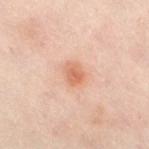Case summary:
• illumination: cross-polarized
• acquisition: total-body-photography crop, ~15 mm field of view
• body site: the right thigh
• subject: female, aged around 55
• lesion diameter: ≈2.5 mm